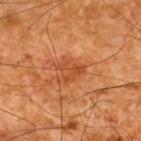No biopsy was performed on this lesion — it was imaged during a full skin examination and was not determined to be concerning.
Located on the upper back.
The lesion-visualizer software estimated a lesion area of about 5.5 mm², an eccentricity of roughly 0.65, and two-axis asymmetry of about 0.4. The software also gave an average lesion color of about L≈39 a*≈25 b*≈34 (CIELAB), roughly 7 lightness units darker than nearby skin, and a normalized lesion–skin contrast near 6. The software also gave border irregularity of about 4 on a 0–10 scale, internal color variation of about 2.5 on a 0–10 scale, and a peripheral color-asymmetry measure near 1. It also reported a nevus-likeness score of about 5/100 and a detector confidence of about 100 out of 100 that the crop contains a lesion.
Captured under cross-polarized illumination.
The patient is a male aged 63–67.
Cropped from a whole-body photographic skin survey; the tile spans about 15 mm.
Measured at roughly 3.5 mm in maximum diameter.